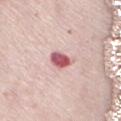No biopsy was performed on this lesion — it was imaged during a full skin examination and was not determined to be concerning.
The patient is a female aged approximately 70.
The lesion is on the front of the torso.
A 15 mm close-up extracted from a 3D total-body photography capture.